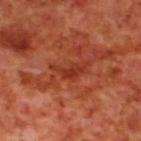  biopsy_status: not biopsied; imaged during a skin examination
  lesion_size:
    long_diameter_mm_approx: 4.0
  automated_metrics:
    cielab_L: 36
    cielab_a: 32
    cielab_b: 35
    vs_skin_darker_L: 8.0
    vs_skin_contrast_norm: 7.0
    border_irregularity_0_10: 7.0
    color_variation_0_10: 1.5
    peripheral_color_asymmetry: 0.5
    nevus_likeness_0_100: 0
    lesion_detection_confidence_0_100: 100
  image:
    source: total-body photography crop
    field_of_view_mm: 15
  patient:
    sex: male
    age_approx: 70
  site: upper back